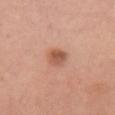Case summary:
- follow-up — no biopsy performed (imaged during a skin exam)
- illumination — white-light
- image source — ~15 mm crop, total-body skin-cancer survey
- anatomic site — the back
- subject — female, aged around 35
- size — ~2.5 mm (longest diameter)